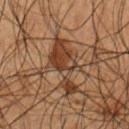The lesion was photographed on a routine skin check and not biopsied; there is no pathology result.
A roughly 15 mm field-of-view crop from a total-body skin photograph.
A male subject roughly 50 years of age.
Captured under cross-polarized illumination.
From the right upper arm.